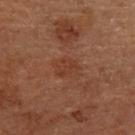<case>
<biopsy_status>not biopsied; imaged during a skin examination</biopsy_status>
<patient>
  <sex>female</sex>
  <age_approx>65</age_approx>
</patient>
<site>left arm</site>
<image>
  <source>total-body photography crop</source>
  <field_of_view_mm>15</field_of_view_mm>
</image>
<lighting>cross-polarized</lighting>
</case>